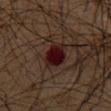Case summary:
• site: the chest
• image: total-body-photography crop, ~15 mm field of view
• subject: male, aged around 65
• automated lesion analysis: a lesion color around L≈12 a*≈19 b*≈13 in CIELAB and a lesion–skin lightness drop of about 11; a classifier nevus-likeness of about 0/100 and a detector confidence of about 100 out of 100 that the crop contains a lesion